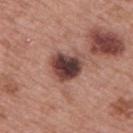The lesion was tiled from a total-body skin photograph and was not biopsied. The tile uses white-light illumination. Approximately 4 mm at its widest. The lesion is located on the upper back. Automated tile analysis of the lesion measured an average lesion color of about L≈40 a*≈21 b*≈21 (CIELAB) and a normalized lesion–skin contrast near 14. And it measured border irregularity of about 3 on a 0–10 scale, a within-lesion color-variation index near 7.5/10, and a peripheral color-asymmetry measure near 2. And it measured an automated nevus-likeness rating near 5 out of 100 and a detector confidence of about 100 out of 100 that the crop contains a lesion. A roughly 15 mm field-of-view crop from a total-body skin photograph. A male patient, aged approximately 65.